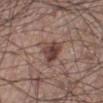This is a white-light tile.
A male subject aged around 60.
A close-up tile cropped from a whole-body skin photograph, about 15 mm across.
The lesion is located on the left lower leg.